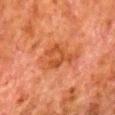The lesion was photographed on a routine skin check and not biopsied; there is no pathology result.
A male patient, roughly 80 years of age.
The lesion's longest dimension is about 6 mm.
Imaged with cross-polarized lighting.
Located on the right lower leg.
A roughly 15 mm field-of-view crop from a total-body skin photograph.